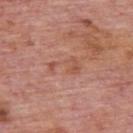The lesion was tiled from a total-body skin photograph and was not biopsied.